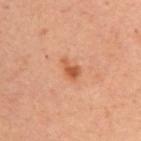| field | value |
|---|---|
| tile lighting | cross-polarized |
| size | ≈3 mm |
| anatomic site | the left upper arm |
| patient | female, approximately 55 years of age |
| imaging modality | 15 mm crop, total-body photography |
| image-analysis metrics | a mean CIELAB color near L≈48 a*≈23 b*≈33; internal color variation of about 2.5 on a 0–10 scale |